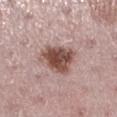Captured during whole-body skin photography for melanoma surveillance; the lesion was not biopsied. The patient is a female approximately 45 years of age. The lesion is on the left lower leg. A close-up tile cropped from a whole-body skin photograph, about 15 mm across. Automated image analysis of the tile measured a mean CIELAB color near L≈49 a*≈21 b*≈23 and about 16 CIELAB-L* units darker than the surrounding skin. It also reported a border-irregularity rating of about 3/10, a within-lesion color-variation index near 5/10, and peripheral color asymmetry of about 1.5. About 4.5 mm across. Captured under white-light illumination.